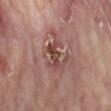biopsy status — catalogued during a skin exam; not biopsied
subject — female, aged 73 to 77
tile lighting — cross-polarized
imaging modality — ~15 mm tile from a whole-body skin photo
body site — the right lower leg
automated metrics — a lesion area of about 5 mm², a shape eccentricity near 0.85, and two-axis asymmetry of about 0.45; a nevus-likeness score of about 0/100
lesion size — about 3.5 mm